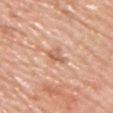Part of a total-body skin-imaging series; this lesion was reviewed on a skin check and was not flagged for biopsy.
Cropped from a total-body skin-imaging series; the visible field is about 15 mm.
The lesion is located on the chest.
About 3 mm across.
This is a white-light tile.
A female patient in their mid- to late 60s.